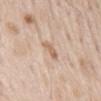No biopsy was performed on this lesion — it was imaged during a full skin examination and was not determined to be concerning. Approximately 2.5 mm at its widest. Located on the mid back. A region of skin cropped from a whole-body photographic capture, roughly 15 mm wide. A male patient, roughly 65 years of age.